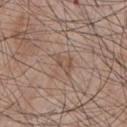Clinical impression: No biopsy was performed on this lesion — it was imaged during a full skin examination and was not determined to be concerning. Image and clinical context: A roughly 15 mm field-of-view crop from a total-body skin photograph. Located on the chest. The patient is a male in their mid- to late 60s.